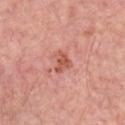{
  "site": "front of the torso",
  "patient": {
    "sex": "male",
    "age_approx": 45
  },
  "lighting": "white-light",
  "image": {
    "source": "total-body photography crop",
    "field_of_view_mm": 15
  },
  "lesion_size": {
    "long_diameter_mm_approx": 3.0
  }
}